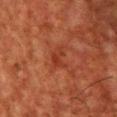Clinical impression:
The lesion was tiled from a total-body skin photograph and was not biopsied.
Image and clinical context:
A 15 mm crop from a total-body photograph taken for skin-cancer surveillance. A male patient, in their 60s. From the chest.